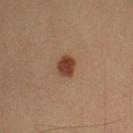Findings:
– follow-up — no biopsy performed (imaged during a skin exam)
– subject — male, aged 38–42
– lesion diameter — ~2.5 mm (longest diameter)
– imaging modality — 15 mm crop, total-body photography
– tile lighting — cross-polarized illumination
– anatomic site — the left upper arm